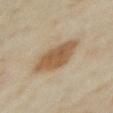Clinical impression: Captured during whole-body skin photography for melanoma surveillance; the lesion was not biopsied. Context: This image is a 15 mm lesion crop taken from a total-body photograph. The recorded lesion diameter is about 5 mm. The lesion is on the left upper arm. A female subject, about 35 years old. The tile uses cross-polarized illumination.